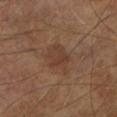<case>
<biopsy_status>not biopsied; imaged during a skin examination</biopsy_status>
<lighting>cross-polarized</lighting>
<image>
  <source>total-body photography crop</source>
  <field_of_view_mm>15</field_of_view_mm>
</image>
<automated_metrics>
  <eccentricity>0.6</eccentricity>
  <shape_asymmetry>0.3</shape_asymmetry>
  <cielab_L>37</cielab_L>
  <cielab_a>18</cielab_a>
  <cielab_b>26</cielab_b>
  <vs_skin_darker_L>6.0</vs_skin_darker_L>
  <color_variation_0_10>2.5</color_variation_0_10>
  <peripheral_color_asymmetry>1.0</peripheral_color_asymmetry>
  <nevus_likeness_0_100>10</nevus_likeness_0_100>
  <lesion_detection_confidence_0_100>100</lesion_detection_confidence_0_100>
</automated_metrics>
<lesion_size>
  <long_diameter_mm_approx>4.0</long_diameter_mm_approx>
</lesion_size>
<patient>
  <sex>male</sex>
  <age_approx>65</age_approx>
</patient>
</case>